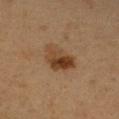A female patient aged 43–47. Imaged with cross-polarized lighting. A roughly 15 mm field-of-view crop from a total-body skin photograph. Located on the right forearm. Measured at roughly 4.5 mm in maximum diameter.